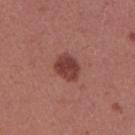Impression:
Recorded during total-body skin imaging; not selected for excision or biopsy.
Background:
From the right upper arm. The total-body-photography lesion software estimated a border-irregularity rating of about 2.5/10, internal color variation of about 3 on a 0–10 scale, and peripheral color asymmetry of about 1. This is a white-light tile. The subject is a female aged around 25. A region of skin cropped from a whole-body photographic capture, roughly 15 mm wide.